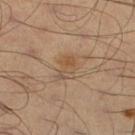No biopsy was performed on this lesion — it was imaged during a full skin examination and was not determined to be concerning. A lesion tile, about 15 mm wide, cut from a 3D total-body photograph. About 3.5 mm across. Captured under cross-polarized illumination. The lesion is on the left leg. A male patient roughly 50 years of age.